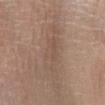image:
  source: total-body photography crop
  field_of_view_mm: 15
site: right lower leg
lesion_size:
  long_diameter_mm_approx: 8.0
lighting: white-light
automated_metrics:
  eccentricity: 0.95
  cielab_L: 52
  cielab_a: 16
  cielab_b: 26
  nevus_likeness_0_100: 0
patient:
  sex: female
  age_approx: 70